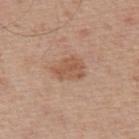Case summary:
- notes — no biopsy performed (imaged during a skin exam)
- size — about 3.5 mm
- imaging modality — ~15 mm tile from a whole-body skin photo
- automated lesion analysis — border irregularity of about 3 on a 0–10 scale, a color-variation rating of about 2.5/10, and radial color variation of about 1; an automated nevus-likeness rating near 15 out of 100 and lesion-presence confidence of about 100/100
- anatomic site — the upper back
- subject — male, aged around 65
- illumination — white-light